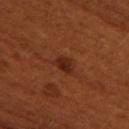Q: What is the lesion's diameter?
A: ≈2.5 mm
Q: What kind of image is this?
A: ~15 mm tile from a whole-body skin photo
Q: Patient demographics?
A: female, approximately 50 years of age
Q: How was the tile lit?
A: cross-polarized